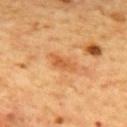No biopsy was performed on this lesion — it was imaged during a full skin examination and was not determined to be concerning.
Longest diameter approximately 3.5 mm.
The tile uses cross-polarized illumination.
The subject is a male in their 60s.
On the mid back.
A 15 mm close-up extracted from a 3D total-body photography capture.